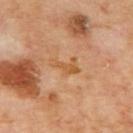Q: Was a biopsy performed?
A: catalogued during a skin exam; not biopsied
Q: Who is the patient?
A: male, roughly 70 years of age
Q: What is the lesion's diameter?
A: about 3.5 mm
Q: How was the tile lit?
A: cross-polarized illumination
Q: What kind of image is this?
A: ~15 mm crop, total-body skin-cancer survey
Q: Where on the body is the lesion?
A: the mid back